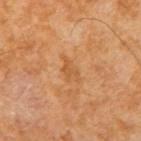biopsy status — imaged on a skin check; not biopsied
TBP lesion metrics — a footprint of about 4 mm², a shape eccentricity near 0.85, and a shape-asymmetry score of about 0.4 (0 = symmetric); a lesion–skin lightness drop of about 7 and a lesion-to-skin contrast of about 5 (normalized; higher = more distinct); a border-irregularity index near 4/10, a color-variation rating of about 2/10, and a peripheral color-asymmetry measure near 1; a nevus-likeness score of about 0/100 and a lesion-detection confidence of about 100/100
acquisition — 15 mm crop, total-body photography
tile lighting — cross-polarized
subject — aged approximately 65
lesion size — about 3 mm
site — the right upper arm